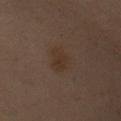The lesion was tiled from a total-body skin photograph and was not biopsied. Approximately 3.5 mm at its widest. This is a cross-polarized tile. A 15 mm crop from a total-body photograph taken for skin-cancer surveillance. On the left upper arm. A female patient aged approximately 55.